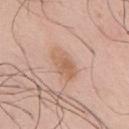{
  "biopsy_status": "not biopsied; imaged during a skin examination",
  "patient": {
    "sex": "male",
    "age_approx": 40
  },
  "lesion_size": {
    "long_diameter_mm_approx": 4.5
  },
  "lighting": "white-light",
  "image": {
    "source": "total-body photography crop",
    "field_of_view_mm": 15
  },
  "site": "mid back"
}